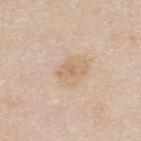- workup · total-body-photography surveillance lesion; no biopsy
- site · the upper back
- subject · male, in their mid-30s
- acquisition · ~15 mm crop, total-body skin-cancer survey